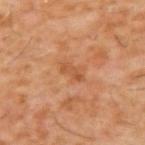The lesion was tiled from a total-body skin photograph and was not biopsied. A 15 mm crop from a total-body photograph taken for skin-cancer surveillance. A male patient roughly 60 years of age. Located on the upper back.